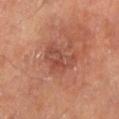<lesion>
  <biopsy_status>not biopsied; imaged during a skin examination</biopsy_status>
  <patient>
    <sex>male</sex>
    <age_approx>65</age_approx>
  </patient>
  <automated_metrics>
    <area_mm2_approx>8.5</area_mm2_approx>
    <eccentricity>0.85</eccentricity>
    <shape_asymmetry>0.4</shape_asymmetry>
  </automated_metrics>
  <site>right lower leg</site>
  <lighting>cross-polarized</lighting>
  <image>
    <source>total-body photography crop</source>
    <field_of_view_mm>15</field_of_view_mm>
  </image>
</lesion>